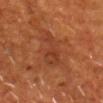Captured during whole-body skin photography for melanoma surveillance; the lesion was not biopsied.
A lesion tile, about 15 mm wide, cut from a 3D total-body photograph.
An algorithmic analysis of the crop reported an area of roughly 8.5 mm² and an eccentricity of roughly 0.95. And it measured lesion-presence confidence of about 100/100.
The subject is a male in their mid- to late 50s.
The lesion is on the chest.